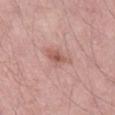{
  "biopsy_status": "not biopsied; imaged during a skin examination",
  "patient": {
    "sex": "male",
    "age_approx": 55
  },
  "site": "leg",
  "lighting": "white-light",
  "automated_metrics": {
    "area_mm2_approx": 4.5,
    "eccentricity": 0.8,
    "shape_asymmetry": 0.35,
    "nevus_likeness_0_100": 10
  },
  "image": {
    "source": "total-body photography crop",
    "field_of_view_mm": 15
  },
  "lesion_size": {
    "long_diameter_mm_approx": 3.0
  }
}